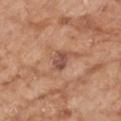Impression:
This lesion was catalogued during total-body skin photography and was not selected for biopsy.
Clinical summary:
The lesion-visualizer software estimated a lesion area of about 4 mm². On the right forearm. Cropped from a total-body skin-imaging series; the visible field is about 15 mm. A female subject, aged 73 to 77.